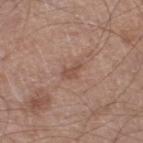<tbp_lesion>
  <biopsy_status>not biopsied; imaged during a skin examination</biopsy_status>
  <patient>
    <sex>male</sex>
    <age_approx>60</age_approx>
  </patient>
  <lesion_size>
    <long_diameter_mm_approx>2.5</long_diameter_mm_approx>
  </lesion_size>
  <site>right lower leg</site>
  <lighting>white-light</lighting>
  <image>
    <source>total-body photography crop</source>
    <field_of_view_mm>15</field_of_view_mm>
  </image>
</tbp_lesion>